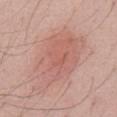The lesion was photographed on a routine skin check and not biopsied; there is no pathology result. A 15 mm close-up extracted from a 3D total-body photography capture. On the abdomen. A male subject about 55 years old.